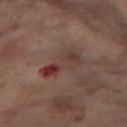Notes:
* notes · no biopsy performed (imaged during a skin exam)
* size · ≈5.5 mm
* tile lighting · cross-polarized
* location · the right thigh
* image · ~15 mm tile from a whole-body skin photo
* subject · female, roughly 55 years of age